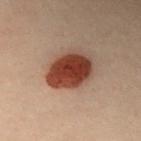biopsy_status: not biopsied; imaged during a skin examination
patient:
  sex: female
  age_approx: 30
lesion_size:
  long_diameter_mm_approx: 5.0
site: chest
image:
  source: total-body photography crop
  field_of_view_mm: 15
lighting: cross-polarized